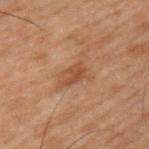Imaged with cross-polarized lighting.
A region of skin cropped from a whole-body photographic capture, roughly 15 mm wide.
Located on the left upper arm.
The patient is a male approximately 70 years of age.